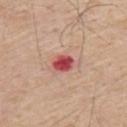{"biopsy_status": "not biopsied; imaged during a skin examination", "lighting": "white-light", "site": "upper back", "image": {"source": "total-body photography crop", "field_of_view_mm": 15}, "lesion_size": {"long_diameter_mm_approx": 3.0}, "automated_metrics": {"area_mm2_approx": 5.0, "eccentricity": 0.65, "shape_asymmetry": 0.15, "cielab_L": 51, "cielab_a": 36, "cielab_b": 26, "vs_skin_darker_L": 16.0, "border_irregularity_0_10": 1.5, "color_variation_0_10": 3.5}, "patient": {"sex": "male", "age_approx": 55}}